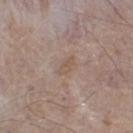• notes — catalogued during a skin exam; not biopsied
• patient — male, aged approximately 65
• lesion size — ≈2.5 mm
• image-analysis metrics — a lesion area of about 3 mm², a shape eccentricity near 0.85, and two-axis asymmetry of about 0.3; an average lesion color of about L≈54 a*≈15 b*≈25 (CIELAB), a lesion–skin lightness drop of about 6, and a normalized border contrast of about 5; a color-variation rating of about 0/10 and a peripheral color-asymmetry measure near 0; a classifier nevus-likeness of about 0/100 and a detector confidence of about 100 out of 100 that the crop contains a lesion
• site — the left thigh
• image source — ~15 mm tile from a whole-body skin photo